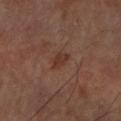Q: Was this lesion biopsied?
A: catalogued during a skin exam; not biopsied
Q: What lighting was used for the tile?
A: cross-polarized
Q: Automated lesion metrics?
A: a lesion–skin lightness drop of about 7; a border-irregularity index near 3.5/10 and a color-variation rating of about 1/10; a nevus-likeness score of about 40/100 and a lesion-detection confidence of about 100/100
Q: Who is the patient?
A: male, aged 68–72
Q: How large is the lesion?
A: ≈2.5 mm
Q: What kind of image is this?
A: total-body-photography crop, ~15 mm field of view
Q: Where on the body is the lesion?
A: the right forearm